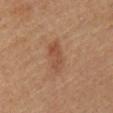The lesion was photographed on a routine skin check and not biopsied; there is no pathology result. The lesion is located on the mid back. A 15 mm close-up tile from a total-body photography series done for melanoma screening. About 4 mm across. Captured under cross-polarized illumination. A male subject about 60 years old.